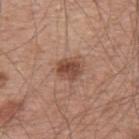The lesion was photographed on a routine skin check and not biopsied; there is no pathology result.
On the upper back.
Imaged with white-light lighting.
This image is a 15 mm lesion crop taken from a total-body photograph.
Measured at roughly 3 mm in maximum diameter.
Automated tile analysis of the lesion measured border irregularity of about 1.5 on a 0–10 scale and internal color variation of about 4.5 on a 0–10 scale. And it measured a detector confidence of about 100 out of 100 that the crop contains a lesion.
A male patient aged 53–57.